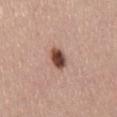No biopsy was performed on this lesion — it was imaged during a full skin examination and was not determined to be concerning.
The subject is a male in their 60s.
A 15 mm close-up extracted from a 3D total-body photography capture.
Located on the mid back.